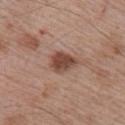Captured during whole-body skin photography for melanoma surveillance; the lesion was not biopsied.
Approximately 3.5 mm at its widest.
A 15 mm close-up extracted from a 3D total-body photography capture.
A male patient, about 65 years old.
Captured under white-light illumination.
On the right upper arm.